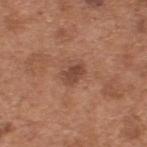| feature | finding |
|---|---|
| workup | total-body-photography surveillance lesion; no biopsy |